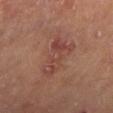The lesion was photographed on a routine skin check and not biopsied; there is no pathology result.
The tile uses cross-polarized illumination.
A close-up tile cropped from a whole-body skin photograph, about 15 mm across.
Located on the right lower leg.
A male subject in their mid- to late 60s.
About 5 mm across.